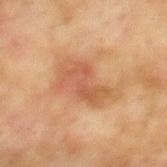Q: Lesion location?
A: the mid back
Q: What kind of image is this?
A: ~15 mm crop, total-body skin-cancer survey
Q: Patient demographics?
A: male, approximately 75 years of age
Q: What did automated image analysis measure?
A: a shape eccentricity near 0.85 and two-axis asymmetry of about 0.35; a mean CIELAB color near L≈48 a*≈20 b*≈30, a lesion–skin lightness drop of about 8, and a normalized border contrast of about 6
Q: Illumination type?
A: cross-polarized
Q: How large is the lesion?
A: ≈6.5 mm